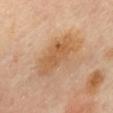Cropped from a total-body skin-imaging series; the visible field is about 15 mm. The total-body-photography lesion software estimated a color-variation rating of about 3.5/10 and peripheral color asymmetry of about 1. The lesion is on the back. A female subject aged approximately 60. Longest diameter approximately 6 mm.